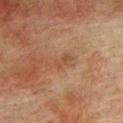Notes:
* biopsy status — imaged on a skin check; not biopsied
* tile lighting — cross-polarized
* patient — male, aged 73–77
* size — ≈3 mm
* acquisition — ~15 mm crop, total-body skin-cancer survey
* body site — the upper back